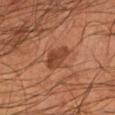Q: Is there a histopathology result?
A: no biopsy performed (imaged during a skin exam)
Q: How was this image acquired?
A: ~15 mm tile from a whole-body skin photo
Q: Lesion location?
A: the left lower leg
Q: Automated lesion metrics?
A: a lesion area of about 6 mm², an eccentricity of roughly 0.75, and two-axis asymmetry of about 0.3; a lesion color around L≈39 a*≈23 b*≈31 in CIELAB, roughly 9 lightness units darker than nearby skin, and a normalized lesion–skin contrast near 8
Q: What lighting was used for the tile?
A: cross-polarized illumination
Q: What are the patient's age and sex?
A: male, in their mid- to late 50s
Q: What is the lesion's diameter?
A: about 3.5 mm